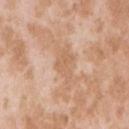Findings:
- follow-up — total-body-photography surveillance lesion; no biopsy
- automated metrics — an area of roughly 6 mm² and an eccentricity of roughly 0.75; an average lesion color of about L≈63 a*≈19 b*≈34 (CIELAB), a lesion–skin lightness drop of about 6, and a normalized border contrast of about 4.5; a within-lesion color-variation index near 1.5/10 and radial color variation of about 0.5; a nevus-likeness score of about 0/100 and a lesion-detection confidence of about 100/100
- image source — ~15 mm tile from a whole-body skin photo
- patient — female, aged approximately 25
- body site — the right upper arm